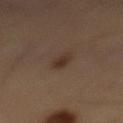Case summary:
- workup — total-body-photography surveillance lesion; no biopsy
- image source — total-body-photography crop, ~15 mm field of view
- patient — male, in their mid-50s
- anatomic site — the mid back